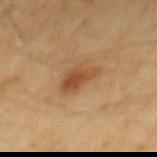Recorded during total-body skin imaging; not selected for excision or biopsy. Measured at roughly 4 mm in maximum diameter. The lesion is located on the mid back. This is a cross-polarized tile. Cropped from a whole-body photographic skin survey; the tile spans about 15 mm. The total-body-photography lesion software estimated an area of roughly 6.5 mm², an eccentricity of roughly 0.8, and a symmetry-axis asymmetry near 0.35. The analysis additionally found a lesion color around L≈40 a*≈17 b*≈31 in CIELAB and about 8 CIELAB-L* units darker than the surrounding skin. The software also gave border irregularity of about 3.5 on a 0–10 scale and radial color variation of about 1.5. And it measured lesion-presence confidence of about 100/100. A male subject, approximately 60 years of age.